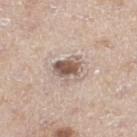Image and clinical context: Approximately 3.5 mm at its widest. The lesion is on the left thigh. A 15 mm crop from a total-body photograph taken for skin-cancer surveillance. Automated tile analysis of the lesion measured an area of roughly 7 mm² and a shape-asymmetry score of about 0.2 (0 = symmetric). And it measured a mean CIELAB color near L≈57 a*≈15 b*≈24, roughly 15 lightness units darker than nearby skin, and a lesion-to-skin contrast of about 9.5 (normalized; higher = more distinct). The analysis additionally found a border-irregularity rating of about 2.5/10, a within-lesion color-variation index near 6/10, and peripheral color asymmetry of about 2. A male subject in their mid- to late 60s. Captured under white-light illumination.